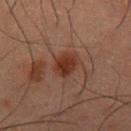notes = no biopsy performed (imaged during a skin exam) | patient = male, approximately 50 years of age | imaging modality = ~15 mm crop, total-body skin-cancer survey | location = the front of the torso | size = about 3 mm | tile lighting = cross-polarized illumination.